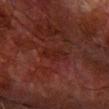Captured during whole-body skin photography for melanoma surveillance; the lesion was not biopsied.
The patient is a male aged around 80.
A region of skin cropped from a whole-body photographic capture, roughly 15 mm wide.
The lesion is on the right forearm.
Longest diameter approximately 3.5 mm.
Automated tile analysis of the lesion measured an area of roughly 4.5 mm², an eccentricity of roughly 0.9, and a shape-asymmetry score of about 0.35 (0 = symmetric). The software also gave a lesion color around L≈17 a*≈21 b*≈20 in CIELAB and a lesion-to-skin contrast of about 5 (normalized; higher = more distinct). It also reported border irregularity of about 4 on a 0–10 scale, a color-variation rating of about 1/10, and a peripheral color-asymmetry measure near 0. The analysis additionally found a lesion-detection confidence of about 95/100.